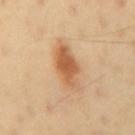• follow-up — catalogued during a skin exam; not biopsied
• site — the mid back
• subject — male, in their 40s
• size — about 5.5 mm
• image source — ~15 mm tile from a whole-body skin photo